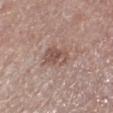<lesion>
<biopsy_status>not biopsied; imaged during a skin examination</biopsy_status>
<lesion_size>
  <long_diameter_mm_approx>3.5</long_diameter_mm_approx>
</lesion_size>
<lighting>white-light</lighting>
<site>right lower leg</site>
<patient>
  <sex>male</sex>
  <age_approx>75</age_approx>
</patient>
<image>
  <source>total-body photography crop</source>
  <field_of_view_mm>15</field_of_view_mm>
</image>
</lesion>